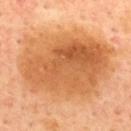The lesion was tiled from a total-body skin photograph and was not biopsied.
Located on the upper back.
The total-body-photography lesion software estimated a shape eccentricity near 0.65. It also reported border irregularity of about 2.5 on a 0–10 scale and internal color variation of about 6.5 on a 0–10 scale. The software also gave a nevus-likeness score of about 70/100 and a detector confidence of about 100 out of 100 that the crop contains a lesion.
A male subject, roughly 65 years of age.
A lesion tile, about 15 mm wide, cut from a 3D total-body photograph.